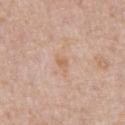| key | value |
|---|---|
| follow-up | catalogued during a skin exam; not biopsied |
| image-analysis metrics | an area of roughly 2.5 mm² and an eccentricity of roughly 0.8; border irregularity of about 3.5 on a 0–10 scale, a color-variation rating of about 1/10, and radial color variation of about 0.5 |
| size | about 2.5 mm |
| imaging modality | 15 mm crop, total-body photography |
| anatomic site | the abdomen |
| subject | male, aged around 60 |
| tile lighting | white-light illumination |